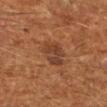The lesion was tiled from a total-body skin photograph and was not biopsied. Captured under cross-polarized illumination. Longest diameter approximately 4 mm. A male patient in their 60s. A 15 mm crop from a total-body photograph taken for skin-cancer surveillance. Located on the right upper arm.